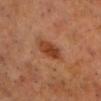A male patient aged approximately 65. Automated tile analysis of the lesion measured a mean CIELAB color near L≈38 a*≈26 b*≈34, roughly 11 lightness units darker than nearby skin, and a normalized border contrast of about 9.5. The analysis additionally found a color-variation rating of about 1/10 and a peripheral color-asymmetry measure near 0.5. Captured under cross-polarized illumination. Located on the head or neck. Cropped from a total-body skin-imaging series; the visible field is about 15 mm.